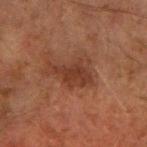{
  "biopsy_status": "not biopsied; imaged during a skin examination",
  "patient": {
    "sex": "male",
    "age_approx": 60
  },
  "image": {
    "source": "total-body photography crop",
    "field_of_view_mm": 15
  },
  "site": "right forearm",
  "lighting": "cross-polarized",
  "lesion_size": {
    "long_diameter_mm_approx": 5.0
  }
}